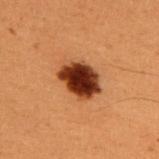No biopsy was performed on this lesion — it was imaged during a full skin examination and was not determined to be concerning. The lesion's longest dimension is about 4.5 mm. The tile uses cross-polarized illumination. A male patient in their 50s. Cropped from a whole-body photographic skin survey; the tile spans about 15 mm. On the upper back. An algorithmic analysis of the crop reported a mean CIELAB color near L≈29 a*≈23 b*≈29 and roughly 19 lightness units darker than nearby skin. And it measured a border-irregularity index near 1.5/10 and a peripheral color-asymmetry measure near 2. The analysis additionally found a lesion-detection confidence of about 100/100.